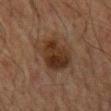Captured during whole-body skin photography for melanoma surveillance; the lesion was not biopsied. The subject is a male roughly 65 years of age. Longest diameter approximately 6 mm. The lesion is located on the mid back. Captured under cross-polarized illumination. An algorithmic analysis of the crop reported an automated nevus-likeness rating near 45 out of 100. A 15 mm crop from a total-body photograph taken for skin-cancer surveillance.